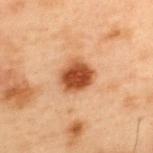Captured during whole-body skin photography for melanoma surveillance; the lesion was not biopsied.
A lesion tile, about 15 mm wide, cut from a 3D total-body photograph.
From the upper back.
A male patient roughly 55 years of age.
The lesion-visualizer software estimated a footprint of about 10 mm² and an outline eccentricity of about 0.55 (0 = round, 1 = elongated). It also reported a lesion color around L≈42 a*≈24 b*≈34 in CIELAB and a lesion–skin lightness drop of about 15. The analysis additionally found an automated nevus-likeness rating near 100 out of 100.
The tile uses cross-polarized illumination.